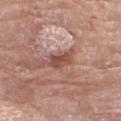Assessment:
The lesion was tiled from a total-body skin photograph and was not biopsied.
Background:
The subject is a female aged 73 to 77. Longest diameter approximately 3 mm. On the left lower leg. A lesion tile, about 15 mm wide, cut from a 3D total-body photograph. The tile uses white-light illumination.